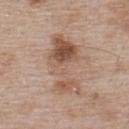No biopsy was performed on this lesion — it was imaged during a full skin examination and was not determined to be concerning. The lesion-visualizer software estimated an area of roughly 21 mm² and an eccentricity of roughly 0.9. The software also gave a mean CIELAB color near L≈55 a*≈18 b*≈29, a lesion–skin lightness drop of about 10, and a normalized border contrast of about 7.5. And it measured border irregularity of about 6 on a 0–10 scale, a color-variation rating of about 9/10, and peripheral color asymmetry of about 3.5. And it measured an automated nevus-likeness rating near 65 out of 100 and a lesion-detection confidence of about 100/100. The patient is a male approximately 55 years of age. Cropped from a total-body skin-imaging series; the visible field is about 15 mm. The lesion is on the upper back.